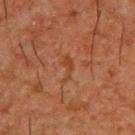notes: total-body-photography surveillance lesion; no biopsy | size: ≈3 mm | subject: male, aged 48 to 52 | tile lighting: cross-polarized | body site: the upper back | image: ~15 mm tile from a whole-body skin photo.